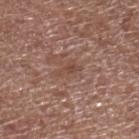{"biopsy_status": "not biopsied; imaged during a skin examination", "lesion_size": {"long_diameter_mm_approx": 2.5}, "site": "right lower leg", "patient": {"sex": "male", "age_approx": 75}, "automated_metrics": {"eccentricity": 0.9, "shape_asymmetry": 0.5, "cielab_L": 46, "cielab_a": 20, "cielab_b": 26, "vs_skin_darker_L": 7.0, "vs_skin_contrast_norm": 5.5, "border_irregularity_0_10": 5.0, "color_variation_0_10": 0.5, "peripheral_color_asymmetry": 0.0}, "image": {"source": "total-body photography crop", "field_of_view_mm": 15}, "lighting": "white-light"}